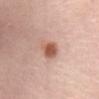{"biopsy_status": "not biopsied; imaged during a skin examination", "patient": {"sex": "female", "age_approx": 65}, "lesion_size": {"long_diameter_mm_approx": 3.5}, "image": {"source": "total-body photography crop", "field_of_view_mm": 15}, "site": "abdomen"}